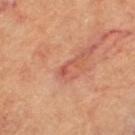Clinical impression: The lesion was photographed on a routine skin check and not biopsied; there is no pathology result. Image and clinical context: The total-body-photography lesion software estimated an area of roughly 2 mm², an outline eccentricity of about 0.9 (0 = round, 1 = elongated), and two-axis asymmetry of about 0.45. The software also gave a normalized lesion–skin contrast near 5.5. It also reported a nevus-likeness score of about 0/100 and a lesion-detection confidence of about 95/100. The lesion is located on the left thigh. The recorded lesion diameter is about 2.5 mm. A female subject roughly 60 years of age. This is a cross-polarized tile. Cropped from a whole-body photographic skin survey; the tile spans about 15 mm.